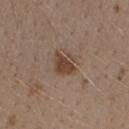Acquisition and patient details: About 3 mm across. This is a white-light tile. A 15 mm close-up tile from a total-body photography series done for melanoma screening. Located on the right forearm. A female patient aged approximately 30.